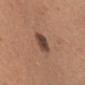Captured during whole-body skin photography for melanoma surveillance; the lesion was not biopsied. The lesion is on the head or neck. This image is a 15 mm lesion crop taken from a total-body photograph. Measured at roughly 2.5 mm in maximum diameter. A female patient aged 63–67. An algorithmic analysis of the crop reported a lesion area of about 5.5 mm² and two-axis asymmetry of about 0.15. The software also gave a classifier nevus-likeness of about 75/100 and lesion-presence confidence of about 100/100.